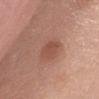<record>
  <site>abdomen</site>
  <lighting>white-light</lighting>
  <patient>
    <sex>female</sex>
    <age_approx>60</age_approx>
  </patient>
  <image>
    <source>total-body photography crop</source>
    <field_of_view_mm>15</field_of_view_mm>
  </image>
  <lesion_size>
    <long_diameter_mm_approx>3.5</long_diameter_mm_approx>
  </lesion_size>
</record>